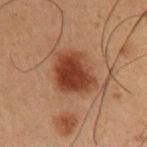Assessment: Part of a total-body skin-imaging series; this lesion was reviewed on a skin check and was not flagged for biopsy. Acquisition and patient details: A male patient in their mid- to late 50s. This image is a 15 mm lesion crop taken from a total-body photograph. An algorithmic analysis of the crop reported a lesion area of about 15 mm², an eccentricity of roughly 0.6, and a shape-asymmetry score of about 0.2 (0 = symmetric). The software also gave an average lesion color of about L≈33 a*≈21 b*≈27 (CIELAB). It also reported a nevus-likeness score of about 100/100 and a lesion-detection confidence of about 100/100. Located on the right upper arm. Captured under cross-polarized illumination.